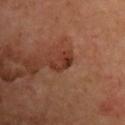* workup — imaged on a skin check; not biopsied
* patient — male, in their mid-60s
* body site — the chest
* illumination — cross-polarized illumination
* TBP lesion metrics — an area of roughly 4 mm², an eccentricity of roughly 0.85, and two-axis asymmetry of about 0.45; a border-irregularity rating of about 6.5/10, a within-lesion color-variation index near 2/10, and peripheral color asymmetry of about 0.5; an automated nevus-likeness rating near 5 out of 100 and lesion-presence confidence of about 100/100
* image — total-body-photography crop, ~15 mm field of view